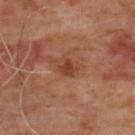Clinical impression: The lesion was photographed on a routine skin check and not biopsied; there is no pathology result. Acquisition and patient details: The lesion is located on the upper back. A male subject aged approximately 65. This image is a 15 mm lesion crop taken from a total-body photograph.